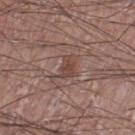biopsy_status: not biopsied; imaged during a skin examination
site: right lower leg
lighting: white-light
patient:
  sex: male
  age_approx: 50
image:
  source: total-body photography crop
  field_of_view_mm: 15
lesion_size:
  long_diameter_mm_approx: 2.5